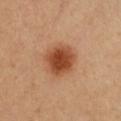Clinical impression: Captured during whole-body skin photography for melanoma surveillance; the lesion was not biopsied. Acquisition and patient details: Located on the chest. Cropped from a whole-body photographic skin survey; the tile spans about 15 mm. A female subject aged 38 to 42.